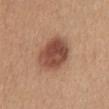The lesion was tiled from a total-body skin photograph and was not biopsied. The lesion's longest dimension is about 5 mm. A female subject, in their mid- to late 30s. A 15 mm close-up tile from a total-body photography series done for melanoma screening. This is a white-light tile. On the chest. The total-body-photography lesion software estimated a lesion area of about 17 mm² and an outline eccentricity of about 0.55 (0 = round, 1 = elongated). And it measured a classifier nevus-likeness of about 100/100 and lesion-presence confidence of about 100/100.